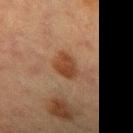Clinical impression: Recorded during total-body skin imaging; not selected for excision or biopsy. Context: A female patient aged 53–57. A roughly 15 mm field-of-view crop from a total-body skin photograph. From the right forearm.